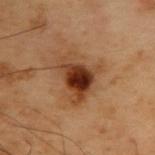biopsy_status: not biopsied; imaged during a skin examination
lesion_size:
  long_diameter_mm_approx: 5.5
automated_metrics:
  area_mm2_approx: 14.0
  eccentricity: 0.7
  shape_asymmetry: 0.4
lighting: cross-polarized
patient:
  sex: male
  age_approx: 55
image:
  source: total-body photography crop
  field_of_view_mm: 15
site: back